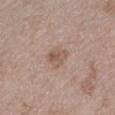Findings:
• follow-up · no biopsy performed (imaged during a skin exam)
• size · ≈2.5 mm
• patient · female, aged 68–72
• acquisition · ~15 mm crop, total-body skin-cancer survey
• location · the left lower leg
• lighting · white-light